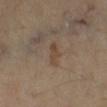patient: male, approximately 65 years of age | imaging modality: ~15 mm tile from a whole-body skin photo | lesion diameter: ~3 mm (longest diameter) | anatomic site: the right lower leg.